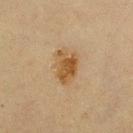notes = catalogued during a skin exam; not biopsied | image = 15 mm crop, total-body photography | lesion diameter = ≈4.5 mm | body site = the left thigh | subject = female, aged 53 to 57.